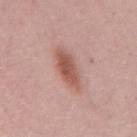Impression:
Imaged during a routine full-body skin examination; the lesion was not biopsied and no histopathology is available.